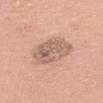Captured during whole-body skin photography for melanoma surveillance; the lesion was not biopsied.
A 15 mm close-up tile from a total-body photography series done for melanoma screening.
A female subject, aged 58 to 62.
On the back.
The recorded lesion diameter is about 6.5 mm.
An algorithmic analysis of the crop reported an average lesion color of about L≈61 a*≈18 b*≈28 (CIELAB), roughly 10 lightness units darker than nearby skin, and a lesion-to-skin contrast of about 6.5 (normalized; higher = more distinct). It also reported border irregularity of about 2.5 on a 0–10 scale, a color-variation rating of about 5.5/10, and a peripheral color-asymmetry measure near 2.
Imaged with white-light lighting.